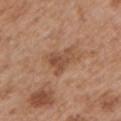biopsy status — no biopsy performed (imaged during a skin exam); lighting — white-light illumination; location — the left upper arm; acquisition — 15 mm crop, total-body photography; lesion size — ~3.5 mm (longest diameter); automated lesion analysis — border irregularity of about 5.5 on a 0–10 scale and a color-variation rating of about 4/10; subject — male, in their mid- to late 50s.